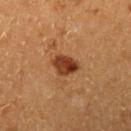On the left upper arm. A female subject, aged approximately 50. Cropped from a total-body skin-imaging series; the visible field is about 15 mm.